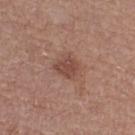Impression:
The lesion was photographed on a routine skin check and not biopsied; there is no pathology result.
Context:
The patient is a female aged 53 to 57. Captured under white-light illumination. Approximately 3 mm at its widest. Located on the right thigh. A 15 mm crop from a total-body photograph taken for skin-cancer surveillance.